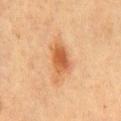{"biopsy_status": "not biopsied; imaged during a skin examination", "site": "front of the torso", "lighting": "cross-polarized", "patient": {"sex": "male", "age_approx": 50}, "automated_metrics": {"cielab_L": 47, "cielab_a": 21, "cielab_b": 34, "vs_skin_darker_L": 10.0, "border_irregularity_0_10": 3.5, "color_variation_0_10": 4.5, "peripheral_color_asymmetry": 1.5}, "image": {"source": "total-body photography crop", "field_of_view_mm": 15}, "lesion_size": {"long_diameter_mm_approx": 5.0}}